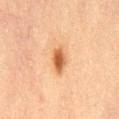The lesion was photographed on a routine skin check and not biopsied; there is no pathology result.
The lesion-visualizer software estimated roughly 13 lightness units darker than nearby skin and a normalized border contrast of about 9. And it measured a nevus-likeness score of about 100/100 and a detector confidence of about 100 out of 100 that the crop contains a lesion.
Approximately 3 mm at its widest.
Imaged with cross-polarized lighting.
Cropped from a total-body skin-imaging series; the visible field is about 15 mm.
A female subject, aged approximately 65.
Located on the lower back.